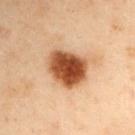Q: Is there a histopathology result?
A: imaged on a skin check; not biopsied
Q: What is the anatomic site?
A: the left upper arm
Q: Patient demographics?
A: male, approximately 55 years of age
Q: What kind of image is this?
A: 15 mm crop, total-body photography